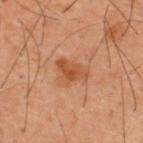Findings:
• biopsy status — total-body-photography surveillance lesion; no biopsy
• site — the back
• image-analysis metrics — internal color variation of about 4 on a 0–10 scale; a lesion-detection confidence of about 100/100
• lighting — cross-polarized
• patient — male, roughly 40 years of age
• image source — ~15 mm tile from a whole-body skin photo